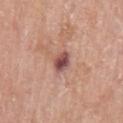On the left upper arm. The recorded lesion diameter is about 3 mm. A 15 mm close-up tile from a total-body photography series done for melanoma screening. The patient is a female roughly 65 years of age.